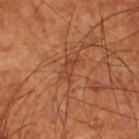Clinical impression: No biopsy was performed on this lesion — it was imaged during a full skin examination and was not determined to be concerning. Image and clinical context: A region of skin cropped from a whole-body photographic capture, roughly 15 mm wide. The recorded lesion diameter is about 3 mm. The lesion is on the right thigh. Automated tile analysis of the lesion measured an eccentricity of roughly 0.9 and two-axis asymmetry of about 0.5. The analysis additionally found a lesion color around L≈41 a*≈25 b*≈32 in CIELAB, a lesion–skin lightness drop of about 6, and a lesion-to-skin contrast of about 5.5 (normalized; higher = more distinct). The software also gave border irregularity of about 6 on a 0–10 scale and radial color variation of about 0. It also reported an automated nevus-likeness rating near 0 out of 100. The tile uses cross-polarized illumination. A male patient about 70 years old.